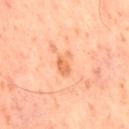{"biopsy_status": "not biopsied; imaged during a skin examination", "image": {"source": "total-body photography crop", "field_of_view_mm": 15}, "lesion_size": {"long_diameter_mm_approx": 3.0}, "patient": {"sex": "male", "age_approx": 65}, "lighting": "cross-polarized"}